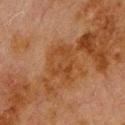follow-up — catalogued during a skin exam; not biopsied
image source — 15 mm crop, total-body photography
subject — male, roughly 80 years of age
automated metrics — an average lesion color of about L≈35 a*≈19 b*≈32 (CIELAB), a lesion–skin lightness drop of about 5, and a lesion-to-skin contrast of about 5.5 (normalized; higher = more distinct); a classifier nevus-likeness of about 0/100 and lesion-presence confidence of about 100/100
anatomic site — the upper back
lighting — cross-polarized illumination